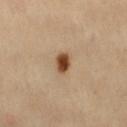Assessment: Imaged during a routine full-body skin examination; the lesion was not biopsied and no histopathology is available. Context: Automated tile analysis of the lesion measured a footprint of about 4.5 mm² and an eccentricity of roughly 0.7. And it measured a border-irregularity rating of about 1.5/10. A 15 mm close-up tile from a total-body photography series done for melanoma screening. A female patient, approximately 60 years of age. This is a cross-polarized tile. The lesion is located on the lower back.